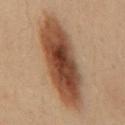Assessment: The lesion was tiled from a total-body skin photograph and was not biopsied. Clinical summary: Captured under cross-polarized illumination. A male patient, aged 28 to 32. Located on the back. Automated tile analysis of the lesion measured a lesion color around L≈37 a*≈17 b*≈26 in CIELAB and a normalized lesion–skin contrast near 11.5. And it measured a border-irregularity index near 2/10, internal color variation of about 6.5 on a 0–10 scale, and radial color variation of about 2. The analysis additionally found a classifier nevus-likeness of about 100/100. A 15 mm close-up extracted from a 3D total-body photography capture.